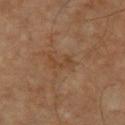| field | value |
|---|---|
| lighting | cross-polarized illumination |
| location | the chest |
| subject | male, about 60 years old |
| lesion size | ≈3 mm |
| imaging modality | total-body-photography crop, ~15 mm field of view |